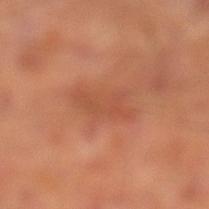Assessment:
Part of a total-body skin-imaging series; this lesion was reviewed on a skin check and was not flagged for biopsy.
Context:
Cropped from a whole-body photographic skin survey; the tile spans about 15 mm. An algorithmic analysis of the crop reported a footprint of about 9.5 mm² and an eccentricity of roughly 0.85. The analysis additionally found border irregularity of about 4 on a 0–10 scale and a peripheral color-asymmetry measure near 0.5. It also reported an automated nevus-likeness rating near 0 out of 100 and a lesion-detection confidence of about 100/100. On the left lower leg. About 5 mm across. The subject is a male aged 63 to 67.